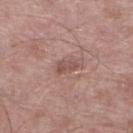The lesion was photographed on a routine skin check and not biopsied; there is no pathology result. Captured under white-light illumination. A male patient in their mid-50s. The lesion's longest dimension is about 2.5 mm. A roughly 15 mm field-of-view crop from a total-body skin photograph. Automated image analysis of the tile measured an average lesion color of about L≈51 a*≈21 b*≈22 (CIELAB), a lesion–skin lightness drop of about 9, and a normalized border contrast of about 6.5. It also reported a border-irregularity index near 3.5/10, a within-lesion color-variation index near 1.5/10, and radial color variation of about 0.5. And it measured an automated nevus-likeness rating near 0 out of 100 and a lesion-detection confidence of about 100/100. The lesion is located on the left lower leg.